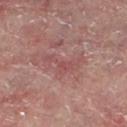This lesion was catalogued during total-body skin photography and was not selected for biopsy. The lesion is located on the left lower leg. The tile uses cross-polarized illumination. Measured at roughly 5 mm in maximum diameter. An algorithmic analysis of the crop reported an outline eccentricity of about 0.9 (0 = round, 1 = elongated) and a symmetry-axis asymmetry near 0.7. And it measured an average lesion color of about L≈43 a*≈22 b*≈19 (CIELAB) and roughly 6 lightness units darker than nearby skin. The analysis additionally found a border-irregularity rating of about 8.5/10, a within-lesion color-variation index near 2/10, and radial color variation of about 0.5. This image is a 15 mm lesion crop taken from a total-body photograph. A male patient, in their 60s.